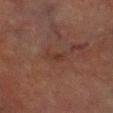workup: catalogued during a skin exam; not biopsied | acquisition: ~15 mm tile from a whole-body skin photo | body site: the leg | patient: male, approximately 70 years of age | automated lesion analysis: a mean CIELAB color near L≈28 a*≈18 b*≈23 and about 4 CIELAB-L* units darker than the surrounding skin; lesion-presence confidence of about 100/100 | diameter: ~3 mm (longest diameter) | illumination: cross-polarized.